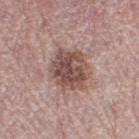Assessment:
No biopsy was performed on this lesion — it was imaged during a full skin examination and was not determined to be concerning.
Acquisition and patient details:
The tile uses white-light illumination. The lesion is located on the right thigh. The total-body-photography lesion software estimated an eccentricity of roughly 0.6. It also reported a lesion color around L≈49 a*≈19 b*≈22 in CIELAB, about 13 CIELAB-L* units darker than the surrounding skin, and a lesion-to-skin contrast of about 9.5 (normalized; higher = more distinct). It also reported border irregularity of about 2 on a 0–10 scale, a color-variation rating of about 5.5/10, and a peripheral color-asymmetry measure near 2. And it measured an automated nevus-likeness rating near 30 out of 100 and a lesion-detection confidence of about 100/100. A roughly 15 mm field-of-view crop from a total-body skin photograph. A female subject about 65 years old.